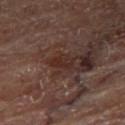biopsy status = no biopsy performed (imaged during a skin exam); location = the right thigh; subject = male, aged 68 to 72; TBP lesion metrics = a lesion area of about 4 mm² and a shape eccentricity near 0.8; imaging modality = 15 mm crop, total-body photography; diameter = about 3 mm; illumination = cross-polarized illumination.